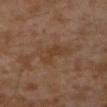No biopsy was performed on this lesion — it was imaged during a full skin examination and was not determined to be concerning. The total-body-photography lesion software estimated an area of roughly 6.5 mm², an eccentricity of roughly 0.9, and two-axis asymmetry of about 0.5. And it measured a lesion color around L≈38 a*≈18 b*≈29 in CIELAB, a lesion–skin lightness drop of about 5, and a normalized border contrast of about 5.5. And it measured a border-irregularity rating of about 5/10 and internal color variation of about 2 on a 0–10 scale. A male patient about 30 years old. Imaged with cross-polarized lighting. Approximately 4.5 mm at its widest. The lesion is on the left lower leg. A region of skin cropped from a whole-body photographic capture, roughly 15 mm wide.